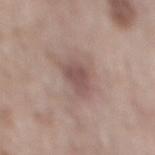Imaged during a routine full-body skin examination; the lesion was not biopsied and no histopathology is available. The patient is a male aged 63–67. From the mid back. Approximately 3.5 mm at its widest. The tile uses white-light illumination. A 15 mm crop from a total-body photograph taken for skin-cancer surveillance.